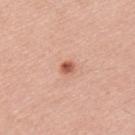Assessment:
Recorded during total-body skin imaging; not selected for excision or biopsy.
Acquisition and patient details:
The lesion is located on the upper back. A male subject about 30 years old. A 15 mm close-up tile from a total-body photography series done for melanoma screening.